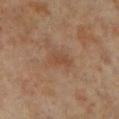{
  "biopsy_status": "not biopsied; imaged during a skin examination"
}